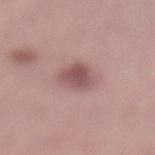Notes:
– follow-up: total-body-photography surveillance lesion; no biopsy
– lesion diameter: ~3.5 mm (longest diameter)
– TBP lesion metrics: a lesion color around L≈52 a*≈21 b*≈19 in CIELAB and a lesion-to-skin contrast of about 7.5 (normalized; higher = more distinct); a border-irregularity index near 2/10 and a peripheral color-asymmetry measure near 1; an automated nevus-likeness rating near 55 out of 100 and a lesion-detection confidence of about 100/100
– patient: female, aged 48 to 52
– tile lighting: white-light illumination
– site: the left lower leg
– acquisition: 15 mm crop, total-body photography